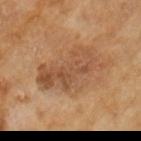Part of a total-body skin-imaging series; this lesion was reviewed on a skin check and was not flagged for biopsy. Longest diameter approximately 7.5 mm. Imaged with cross-polarized lighting. The patient is a male approximately 65 years of age. A lesion tile, about 15 mm wide, cut from a 3D total-body photograph.